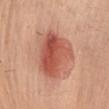Context: The total-body-photography lesion software estimated a border-irregularity rating of about 2.5/10, a within-lesion color-variation index near 8.5/10, and a peripheral color-asymmetry measure near 3. The lesion is on the front of the torso. The subject is a female about 65 years old. Longest diameter approximately 5.5 mm. A region of skin cropped from a whole-body photographic capture, roughly 15 mm wide.